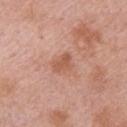workup — no biopsy performed (imaged during a skin exam)
site — the left upper arm
image-analysis metrics — a lesion area of about 4.5 mm² and a shape-asymmetry score of about 0.3 (0 = symmetric); a lesion color around L≈56 a*≈24 b*≈31 in CIELAB, a lesion–skin lightness drop of about 8, and a lesion-to-skin contrast of about 6 (normalized; higher = more distinct); a detector confidence of about 100 out of 100 that the crop contains a lesion
diameter — ~2.5 mm (longest diameter)
subject — male, aged around 55
lighting — white-light
image source — ~15 mm crop, total-body skin-cancer survey